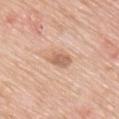Recorded during total-body skin imaging; not selected for excision or biopsy. A male patient aged approximately 80. About 3 mm across. Imaged with white-light lighting. A region of skin cropped from a whole-body photographic capture, roughly 15 mm wide. An algorithmic analysis of the crop reported a footprint of about 5.5 mm², an outline eccentricity of about 0.75 (0 = round, 1 = elongated), and two-axis asymmetry of about 0.2. It also reported a border-irregularity rating of about 2/10, a color-variation rating of about 2.5/10, and radial color variation of about 1. From the mid back.